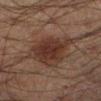notes: catalogued during a skin exam; not biopsied.